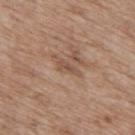This lesion was catalogued during total-body skin photography and was not selected for biopsy. A male subject, about 70 years old. Longest diameter approximately 3 mm. A 15 mm crop from a total-body photograph taken for skin-cancer surveillance. The lesion is located on the mid back. Imaged with white-light lighting.